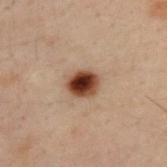Part of a total-body skin-imaging series; this lesion was reviewed on a skin check and was not flagged for biopsy.
A region of skin cropped from a whole-body photographic capture, roughly 15 mm wide.
The total-body-photography lesion software estimated a border-irregularity rating of about 1.5/10, a color-variation rating of about 7.5/10, and radial color variation of about 2. The analysis additionally found a nevus-likeness score of about 100/100.
The lesion is on the upper back.
The patient is a male aged 28 to 32.
Measured at roughly 3.5 mm in maximum diameter.